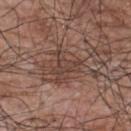<record>
<biopsy_status>not biopsied; imaged during a skin examination</biopsy_status>
<automated_metrics>
  <border_irregularity_0_10>5.5</border_irregularity_0_10>
  <peripheral_color_asymmetry>1.0</peripheral_color_asymmetry>
  <nevus_likeness_0_100>0</nevus_likeness_0_100>
  <lesion_detection_confidence_0_100>70</lesion_detection_confidence_0_100>
</automated_metrics>
<patient>
  <sex>male</sex>
  <age_approx>70</age_approx>
</patient>
<site>upper back</site>
<lesion_size>
  <long_diameter_mm_approx>4.5</long_diameter_mm_approx>
</lesion_size>
<image>
  <source>total-body photography crop</source>
  <field_of_view_mm>15</field_of_view_mm>
</image>
</record>